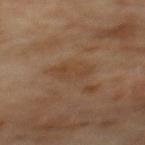  biopsy_status: not biopsied; imaged during a skin examination
  lighting: cross-polarized
  image:
    source: total-body photography crop
    field_of_view_mm: 15
  site: mid back
  lesion_size:
    long_diameter_mm_approx: 4.0
  automated_metrics:
    area_mm2_approx: 7.0
    shape_asymmetry: 0.25
    lesion_detection_confidence_0_100: 100
  patient:
    sex: male
    age_approx: 70